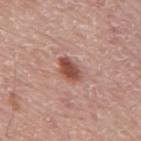This image is a 15 mm lesion crop taken from a total-body photograph. On the mid back. A male subject aged around 75.